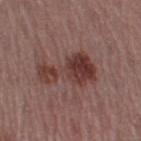<case>
<site>right thigh</site>
<patient>
  <sex>female</sex>
  <age_approx>55</age_approx>
</patient>
<image>
  <source>total-body photography crop</source>
  <field_of_view_mm>15</field_of_view_mm>
</image>
<lighting>white-light</lighting>
<automated_metrics>
  <border_irregularity_0_10>8.0</border_irregularity_0_10>
  <color_variation_0_10>5.5</color_variation_0_10>
</automated_metrics>
<lesion_size>
  <long_diameter_mm_approx>6.5</long_diameter_mm_approx>
</lesion_size>
</case>